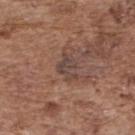Clinical impression:
The lesion was tiled from a total-body skin photograph and was not biopsied.
Context:
From the upper back. Longest diameter approximately 3 mm. A male patient roughly 75 years of age. Captured under white-light illumination. A 15 mm close-up tile from a total-body photography series done for melanoma screening.